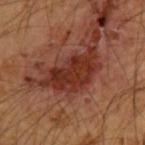Imaged during a routine full-body skin examination; the lesion was not biopsied and no histopathology is available.
Approximately 7 mm at its widest.
Located on the upper back.
A male patient in their mid-50s.
This is a cross-polarized tile.
Cropped from a total-body skin-imaging series; the visible field is about 15 mm.